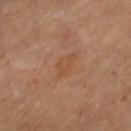Impression: The lesion was tiled from a total-body skin photograph and was not biopsied. Context: Automated image analysis of the tile measured a border-irregularity index near 3/10 and a peripheral color-asymmetry measure near 1. And it measured an automated nevus-likeness rating near 0 out of 100 and a lesion-detection confidence of about 100/100. Cropped from a total-body skin-imaging series; the visible field is about 15 mm. A female subject, roughly 70 years of age. Located on the left thigh. About 4 mm across.